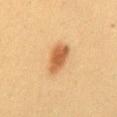No biopsy was performed on this lesion — it was imaged during a full skin examination and was not determined to be concerning. On the mid back. This is a cross-polarized tile. The subject is a female aged 38–42. A close-up tile cropped from a whole-body skin photograph, about 15 mm across. Approximately 4 mm at its widest.